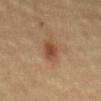Clinical impression: Part of a total-body skin-imaging series; this lesion was reviewed on a skin check and was not flagged for biopsy. Clinical summary: The lesion is located on the abdomen. The subject is a female aged 53 to 57. A region of skin cropped from a whole-body photographic capture, roughly 15 mm wide. Imaged with cross-polarized lighting. The total-body-photography lesion software estimated a border-irregularity index near 2/10 and a color-variation rating of about 3/10. It also reported an automated nevus-likeness rating near 85 out of 100 and a detector confidence of about 100 out of 100 that the crop contains a lesion.